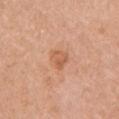Captured during whole-body skin photography for melanoma surveillance; the lesion was not biopsied. A 15 mm close-up tile from a total-body photography series done for melanoma screening. Located on the left upper arm. Automated image analysis of the tile measured an area of roughly 5 mm² and a shape eccentricity near 0.5. And it measured a classifier nevus-likeness of about 5/100 and a lesion-detection confidence of about 100/100. The tile uses white-light illumination. A female patient approximately 60 years of age.